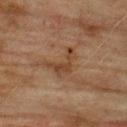Imaged during a routine full-body skin examination; the lesion was not biopsied and no histopathology is available.
A roughly 15 mm field-of-view crop from a total-body skin photograph.
The tile uses cross-polarized illumination.
The patient is a male roughly 75 years of age.
Located on the back.
Automated image analysis of the tile measured a mean CIELAB color near L≈34 a*≈17 b*≈27 and a lesion–skin lightness drop of about 7. The software also gave a border-irregularity rating of about 8.5/10, a color-variation rating of about 1.5/10, and a peripheral color-asymmetry measure near 0.5. The analysis additionally found an automated nevus-likeness rating near 0 out of 100.
Approximately 4 mm at its widest.